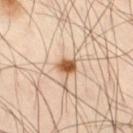Part of a total-body skin-imaging series; this lesion was reviewed on a skin check and was not flagged for biopsy.
A lesion tile, about 15 mm wide, cut from a 3D total-body photograph.
Automated image analysis of the tile measured a shape eccentricity near 0.65. The analysis additionally found a lesion color around L≈58 a*≈21 b*≈36 in CIELAB, about 17 CIELAB-L* units darker than the surrounding skin, and a normalized border contrast of about 11. The analysis additionally found a border-irregularity rating of about 2/10, a within-lesion color-variation index near 4.5/10, and a peripheral color-asymmetry measure near 1.5.
The tile uses cross-polarized illumination.
A male subject, aged around 50.
Measured at roughly 2.5 mm in maximum diameter.
Located on the leg.